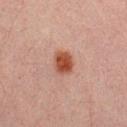Clinical impression:
Part of a total-body skin-imaging series; this lesion was reviewed on a skin check and was not flagged for biopsy.
Acquisition and patient details:
The patient is a male aged around 30. On the chest. Longest diameter approximately 3 mm. Cropped from a total-body skin-imaging series; the visible field is about 15 mm. Captured under cross-polarized illumination.